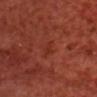Imaged during a routine full-body skin examination; the lesion was not biopsied and no histopathology is available.
The recorded lesion diameter is about 2.5 mm.
The lesion is on the front of the torso.
A lesion tile, about 15 mm wide, cut from a 3D total-body photograph.
This is a cross-polarized tile.
A male subject, aged 68–72.